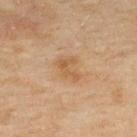The lesion was tiled from a total-body skin photograph and was not biopsied.
A male patient, aged around 45.
Located on the upper back.
A 15 mm close-up tile from a total-body photography series done for melanoma screening.
An algorithmic analysis of the crop reported a lesion area of about 4 mm², an outline eccentricity of about 0.8 (0 = round, 1 = elongated), and a symmetry-axis asymmetry near 0.55. The software also gave a mean CIELAB color near L≈48 a*≈17 b*≈33, roughly 7 lightness units darker than nearby skin, and a normalized border contrast of about 6. It also reported a border-irregularity index near 7/10, a within-lesion color-variation index near 0/10, and a peripheral color-asymmetry measure near 0. The software also gave a classifier nevus-likeness of about 0/100 and a detector confidence of about 100 out of 100 that the crop contains a lesion.
Imaged with cross-polarized lighting.